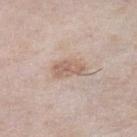The lesion was tiled from a total-body skin photograph and was not biopsied. A 15 mm crop from a total-body photograph taken for skin-cancer surveillance. Longest diameter approximately 4 mm. A female patient, roughly 60 years of age. From the leg. Automated image analysis of the tile measured border irregularity of about 2.5 on a 0–10 scale, internal color variation of about 3 on a 0–10 scale, and a peripheral color-asymmetry measure near 1. Imaged with white-light lighting.